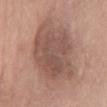This lesion was catalogued during total-body skin photography and was not selected for biopsy.
About 9.5 mm across.
The lesion is located on the abdomen.
Automated image analysis of the tile measured a lesion area of about 44 mm² and an outline eccentricity of about 0.75 (0 = round, 1 = elongated). It also reported internal color variation of about 3.5 on a 0–10 scale and a peripheral color-asymmetry measure near 1.
A region of skin cropped from a whole-body photographic capture, roughly 15 mm wide.
The subject is a female aged 58 to 62.